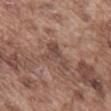Q: Is there a histopathology result?
A: no biopsy performed (imaged during a skin exam)